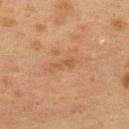biopsy status: imaged on a skin check; not biopsied
image-analysis metrics: a lesion area of about 2.5 mm², an outline eccentricity of about 0.95 (0 = round, 1 = elongated), and two-axis asymmetry of about 0.4; an average lesion color of about L≈45 a*≈18 b*≈31 (CIELAB), about 5 CIELAB-L* units darker than the surrounding skin, and a normalized lesion–skin contrast near 5; border irregularity of about 4.5 on a 0–10 scale; a classifier nevus-likeness of about 0/100 and lesion-presence confidence of about 100/100
imaging modality: ~15 mm crop, total-body skin-cancer survey
lighting: cross-polarized
subject: female, approximately 40 years of age
site: the back